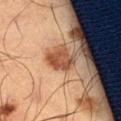A roughly 15 mm field-of-view crop from a total-body skin photograph. A male patient, in their mid-60s. The lesion is located on the right thigh. Captured under cross-polarized illumination. An algorithmic analysis of the crop reported an automated nevus-likeness rating near 85 out of 100 and a detector confidence of about 100 out of 100 that the crop contains a lesion. Approximately 3 mm at its widest.